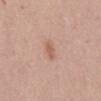This lesion was catalogued during total-body skin photography and was not selected for biopsy. Located on the mid back. Cropped from a total-body skin-imaging series; the visible field is about 15 mm. A female subject, aged 58 to 62.